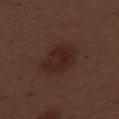Clinical impression:
No biopsy was performed on this lesion — it was imaged during a full skin examination and was not determined to be concerning.
Image and clinical context:
The tile uses white-light illumination. The lesion is located on the right thigh. A 15 mm crop from a total-body photograph taken for skin-cancer surveillance. A male subject roughly 70 years of age.